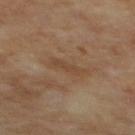This is a cross-polarized tile.
A male subject approximately 70 years of age.
Located on the mid back.
A 15 mm close-up tile from a total-body photography series done for melanoma screening.